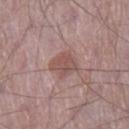{
  "biopsy_status": "not biopsied; imaged during a skin examination",
  "image": {
    "source": "total-body photography crop",
    "field_of_view_mm": 15
  },
  "patient": {
    "sex": "male",
    "age_approx": 65
  },
  "automated_metrics": {
    "area_mm2_approx": 7.5,
    "eccentricity": 0.6,
    "shape_asymmetry": 0.25,
    "border_irregularity_0_10": 2.5,
    "color_variation_0_10": 2.5,
    "peripheral_color_asymmetry": 1.0
  },
  "site": "leg",
  "lighting": "white-light"
}